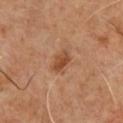Assessment: Part of a total-body skin-imaging series; this lesion was reviewed on a skin check and was not flagged for biopsy. Context: A close-up tile cropped from a whole-body skin photograph, about 15 mm across. Approximately 3 mm at its widest. A male patient, aged 48–52. Imaged with cross-polarized lighting. On the chest. The lesion-visualizer software estimated a footprint of about 4 mm² and an outline eccentricity of about 0.8 (0 = round, 1 = elongated). The analysis additionally found a mean CIELAB color near L≈47 a*≈23 b*≈35 and a normalized lesion–skin contrast near 8. The software also gave lesion-presence confidence of about 100/100.